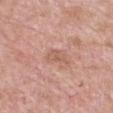  biopsy_status: not biopsied; imaged during a skin examination
  site: chest
  image:
    source: total-body photography crop
    field_of_view_mm: 15
  patient:
    sex: female
    age_approx: 75